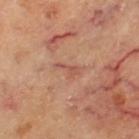biopsy_status: not biopsied; imaged during a skin examination
lighting: cross-polarized
image:
  source: total-body photography crop
  field_of_view_mm: 15
site: leg
automated_metrics:
  cielab_L: 55
  cielab_a: 26
  cielab_b: 30
  vs_skin_darker_L: 8.0
  vs_skin_contrast_norm: 5.5
  peripheral_color_asymmetry: 0.0
lesion_size:
  long_diameter_mm_approx: 3.0
patient:
  sex: female
  age_approx: 60